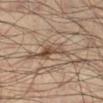Imaged during a routine full-body skin examination; the lesion was not biopsied and no histopathology is available. The patient is a male about 35 years old. The lesion is located on the right lower leg. Cropped from a whole-body photographic skin survey; the tile spans about 15 mm. This is a cross-polarized tile.